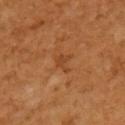Recorded during total-body skin imaging; not selected for excision or biopsy.
A roughly 15 mm field-of-view crop from a total-body skin photograph.
From the left upper arm.
The subject is a female aged 53–57.